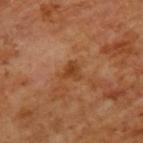{
  "biopsy_status": "not biopsied; imaged during a skin examination",
  "image": {
    "source": "total-body photography crop",
    "field_of_view_mm": 15
  },
  "automated_metrics": {
    "cielab_L": 41,
    "cielab_a": 24,
    "cielab_b": 36,
    "vs_skin_darker_L": 9.0,
    "border_irregularity_0_10": 4.5
  },
  "lesion_size": {
    "long_diameter_mm_approx": 2.5
  },
  "site": "upper back",
  "patient": {
    "sex": "male",
    "age_approx": 65
  },
  "lighting": "cross-polarized"
}